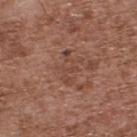Captured during whole-body skin photography for melanoma surveillance; the lesion was not biopsied.
A 15 mm close-up extracted from a 3D total-body photography capture.
The recorded lesion diameter is about 4 mm.
The total-body-photography lesion software estimated a footprint of about 5.5 mm², an outline eccentricity of about 0.9 (0 = round, 1 = elongated), and two-axis asymmetry of about 0.4. The software also gave a color-variation rating of about 2/10 and radial color variation of about 0.5. It also reported a nevus-likeness score of about 0/100.
The lesion is on the upper back.
The patient is a male aged 73–77.
The tile uses white-light illumination.